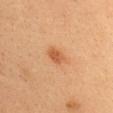| field | value |
|---|---|
| notes | catalogued during a skin exam; not biopsied |
| imaging modality | ~15 mm tile from a whole-body skin photo |
| location | the head or neck |
| tile lighting | cross-polarized |
| subject | female, roughly 35 years of age |